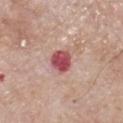Clinical impression: This lesion was catalogued during total-body skin photography and was not selected for biopsy. Clinical summary: Measured at roughly 3 mm in maximum diameter. Captured under white-light illumination. An algorithmic analysis of the crop reported a mean CIELAB color near L≈51 a*≈33 b*≈21, about 16 CIELAB-L* units darker than the surrounding skin, and a normalized border contrast of about 11. The software also gave a border-irregularity rating of about 1.5/10 and internal color variation of about 4 on a 0–10 scale. And it measured a nevus-likeness score of about 0/100 and a lesion-detection confidence of about 100/100. A male subject, aged approximately 65. From the chest. A 15 mm close-up extracted from a 3D total-body photography capture.